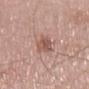This image is a 15 mm lesion crop taken from a total-body photograph. Measured at roughly 4.5 mm in maximum diameter. From the right thigh. A male subject, in their mid-60s. Imaged with white-light lighting.